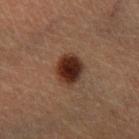<case>
<biopsy_status>not biopsied; imaged during a skin examination</biopsy_status>
<image>
  <source>total-body photography crop</source>
  <field_of_view_mm>15</field_of_view_mm>
</image>
<lighting>cross-polarized</lighting>
<site>right lower leg</site>
<patient>
  <sex>male</sex>
  <age_approx>60</age_approx>
</patient>
<lesion_size>
  <long_diameter_mm_approx>3.5</long_diameter_mm_approx>
</lesion_size>
</case>